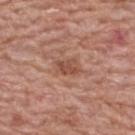notes = imaged on a skin check; not biopsied
tile lighting = white-light
lesion diameter = about 2.5 mm
image source = total-body-photography crop, ~15 mm field of view
site = the upper back
subject = female, aged 58–62
TBP lesion metrics = a nevus-likeness score of about 0/100 and a detector confidence of about 100 out of 100 that the crop contains a lesion